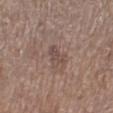Recorded during total-body skin imaging; not selected for excision or biopsy. A region of skin cropped from a whole-body photographic capture, roughly 15 mm wide. The lesion's longest dimension is about 3 mm. The patient is a male aged approximately 65. On the left lower leg. This is a white-light tile.